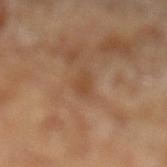Impression: The lesion was photographed on a routine skin check and not biopsied; there is no pathology result. Acquisition and patient details: Automated tile analysis of the lesion measured a footprint of about 4 mm², an outline eccentricity of about 0.9 (0 = round, 1 = elongated), and two-axis asymmetry of about 0.35. It also reported a color-variation rating of about 1/10 and radial color variation of about 0. The analysis additionally found a nevus-likeness score of about 0/100 and a detector confidence of about 100 out of 100 that the crop contains a lesion. From the left lower leg. A 15 mm close-up extracted from a 3D total-body photography capture. A male subject aged 68–72. This is a cross-polarized tile. The lesion's longest dimension is about 3.5 mm.